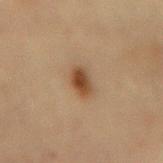<record>
  <biopsy_status>not biopsied; imaged during a skin examination</biopsy_status>
  <site>back</site>
  <automated_metrics>
    <area_mm2_approx>5.0</area_mm2_approx>
    <shape_asymmetry>0.25</shape_asymmetry>
    <cielab_L>40</cielab_L>
    <cielab_a>17</cielab_a>
    <cielab_b>30</cielab_b>
    <vs_skin_darker_L>12.0</vs_skin_darker_L>
    <vs_skin_contrast_norm>9.5</vs_skin_contrast_norm>
    <nevus_likeness_0_100>100</nevus_likeness_0_100>
    <lesion_detection_confidence_0_100>100</lesion_detection_confidence_0_100>
  </automated_metrics>
  <patient>
    <sex>female</sex>
    <age_approx>70</age_approx>
  </patient>
  <lighting>cross-polarized</lighting>
  <image>
    <source>total-body photography crop</source>
    <field_of_view_mm>15</field_of_view_mm>
  </image>
</record>